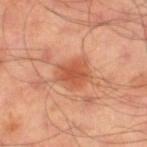Q: Was this lesion biopsied?
A: no biopsy performed (imaged during a skin exam)
Q: What are the patient's age and sex?
A: male, aged around 55
Q: How large is the lesion?
A: about 3.5 mm
Q: What is the anatomic site?
A: the right thigh
Q: What lighting was used for the tile?
A: cross-polarized
Q: What kind of image is this?
A: ~15 mm tile from a whole-body skin photo
Q: What did automated image analysis measure?
A: a lesion color around L≈55 a*≈29 b*≈36 in CIELAB and a normalized border contrast of about 7